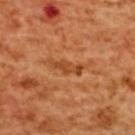The lesion was tiled from a total-body skin photograph and was not biopsied.
The lesion is located on the upper back.
The recorded lesion diameter is about 4 mm.
A 15 mm close-up extracted from a 3D total-body photography capture.
Captured under cross-polarized illumination.
A female subject, approximately 55 years of age.
Automated image analysis of the tile measured a lesion area of about 4 mm², an eccentricity of roughly 0.95, and two-axis asymmetry of about 0.3. The analysis additionally found a lesion–skin lightness drop of about 9 and a normalized lesion–skin contrast near 7. The software also gave a nevus-likeness score of about 0/100 and lesion-presence confidence of about 95/100.